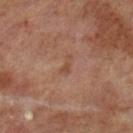Q: Was a biopsy performed?
A: total-body-photography surveillance lesion; no biopsy
Q: What is the anatomic site?
A: the left lower leg
Q: Who is the patient?
A: male, aged around 70
Q: Automated lesion metrics?
A: a mean CIELAB color near L≈46 a*≈20 b*≈29; border irregularity of about 5.5 on a 0–10 scale and radial color variation of about 0; a nevus-likeness score of about 0/100 and lesion-presence confidence of about 100/100
Q: What kind of image is this?
A: ~15 mm tile from a whole-body skin photo